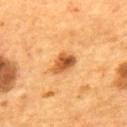{"biopsy_status": "not biopsied; imaged during a skin examination", "lighting": "cross-polarized", "site": "upper back", "image": {"source": "total-body photography crop", "field_of_view_mm": 15}, "lesion_size": {"long_diameter_mm_approx": 3.0}, "automated_metrics": {"area_mm2_approx": 5.5, "eccentricity": 0.7, "shape_asymmetry": 0.25}, "patient": {"sex": "male", "age_approx": 55}}